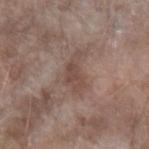Impression: Captured during whole-body skin photography for melanoma surveillance; the lesion was not biopsied. Acquisition and patient details: This is a white-light tile. On the left forearm. A lesion tile, about 15 mm wide, cut from a 3D total-body photograph. A female patient, approximately 75 years of age.